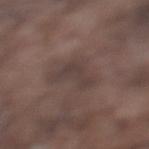Q: Was a biopsy performed?
A: catalogued during a skin exam; not biopsied
Q: What kind of image is this?
A: total-body-photography crop, ~15 mm field of view
Q: Patient demographics?
A: male, aged 73–77
Q: What is the anatomic site?
A: the left lower leg